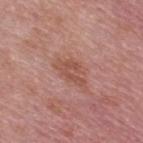<case>
<biopsy_status>not biopsied; imaged during a skin examination</biopsy_status>
<image>
  <source>total-body photography crop</source>
  <field_of_view_mm>15</field_of_view_mm>
</image>
<lesion_size>
  <long_diameter_mm_approx>4.0</long_diameter_mm_approx>
</lesion_size>
<site>upper back</site>
<patient>
  <sex>male</sex>
  <age_approx>55</age_approx>
</patient>
<automated_metrics>
  <area_mm2_approx>5.5</area_mm2_approx>
  <nevus_likeness_0_100>0</nevus_likeness_0_100>
  <lesion_detection_confidence_0_100>100</lesion_detection_confidence_0_100>
</automated_metrics>
</case>